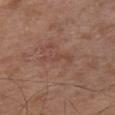Clinical impression: The lesion was photographed on a routine skin check and not biopsied; there is no pathology result. Context: A female patient approximately 65 years of age. The lesion's longest dimension is about 4.5 mm. From the front of the torso. Automated tile analysis of the lesion measured a border-irregularity index near 6.5/10 and internal color variation of about 0.5 on a 0–10 scale. The tile uses white-light illumination. A lesion tile, about 15 mm wide, cut from a 3D total-body photograph.